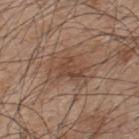| key | value |
|---|---|
| biopsy status | total-body-photography surveillance lesion; no biopsy |
| automated metrics | a border-irregularity index near 5.5/10, a color-variation rating of about 2.5/10, and peripheral color asymmetry of about 1; a nevus-likeness score of about 20/100 |
| imaging modality | ~15 mm tile from a whole-body skin photo |
| patient | male, aged 43 to 47 |
| tile lighting | white-light |
| size | ~4.5 mm (longest diameter) |
| body site | the upper back |